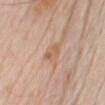follow-up=total-body-photography surveillance lesion; no biopsy
imaging modality=~15 mm tile from a whole-body skin photo
site=the chest
subject=male, aged approximately 80
diameter=about 3 mm
lighting=white-light illumination